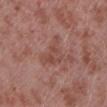notes = no biopsy performed (imaged during a skin exam) | acquisition = ~15 mm crop, total-body skin-cancer survey | patient = male, approximately 55 years of age | tile lighting = white-light illumination | anatomic site = the left lower leg.